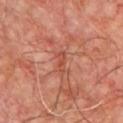follow-up: no biopsy performed (imaged during a skin exam) | patient: male, aged approximately 55 | lighting: cross-polarized illumination | location: the chest | image-analysis metrics: a shape eccentricity near 0.95; a mean CIELAB color near L≈49 a*≈31 b*≈31 and a normalized border contrast of about 5.5; a classifier nevus-likeness of about 0/100 | image source: 15 mm crop, total-body photography.